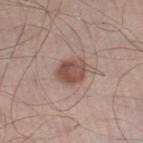biopsy_status: not biopsied; imaged during a skin examination
patient:
  sex: male
  age_approx: 55
lighting: white-light
automated_metrics:
  cielab_L: 50
  cielab_a: 20
  cielab_b: 24
  vs_skin_darker_L: 12.0
  border_irregularity_0_10: 2.0
  color_variation_0_10: 5.0
  nevus_likeness_0_100: 90
  lesion_detection_confidence_0_100: 100
image:
  source: total-body photography crop
  field_of_view_mm: 15
lesion_size:
  long_diameter_mm_approx: 3.5
site: leg